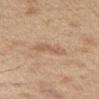No biopsy was performed on this lesion — it was imaged during a full skin examination and was not determined to be concerning.
The lesion is located on the head or neck.
Cropped from a total-body skin-imaging series; the visible field is about 15 mm.
A female patient, in their 40s.